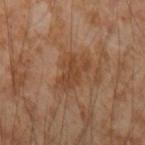Impression:
This lesion was catalogued during total-body skin photography and was not selected for biopsy.
Image and clinical context:
Measured at roughly 4.5 mm in maximum diameter. A male subject, in their mid- to late 50s. Automated image analysis of the tile measured an area of roughly 9 mm², an eccentricity of roughly 0.8, and two-axis asymmetry of about 0.35. The tile uses cross-polarized illumination. The lesion is located on the arm. This image is a 15 mm lesion crop taken from a total-body photograph.